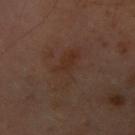Clinical impression:
The lesion was tiled from a total-body skin photograph and was not biopsied.
Acquisition and patient details:
An algorithmic analysis of the crop reported about 3 CIELAB-L* units darker than the surrounding skin and a normalized border contrast of about 5. It also reported border irregularity of about 5 on a 0–10 scale, a color-variation rating of about 3/10, and a peripheral color-asymmetry measure near 1. Imaged with cross-polarized lighting. A female subject aged approximately 60. A 15 mm crop from a total-body photograph taken for skin-cancer surveillance. The recorded lesion diameter is about 5 mm. On the front of the torso.